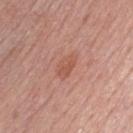Q: Was a biopsy performed?
A: no biopsy performed (imaged during a skin exam)
Q: Patient demographics?
A: male, about 60 years old
Q: Illumination type?
A: white-light
Q: What kind of image is this?
A: 15 mm crop, total-body photography
Q: What is the anatomic site?
A: the chest